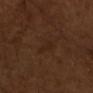Case summary:
* follow-up — imaged on a skin check; not biopsied
* lighting — cross-polarized illumination
* location — the front of the torso
* patient — male, about 45 years old
* lesion diameter — about 2.5 mm
* image — total-body-photography crop, ~15 mm field of view